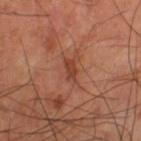Case summary:
• biopsy status · total-body-photography surveillance lesion; no biopsy
• tile lighting · cross-polarized illumination
• patient · male, in their 60s
• diameter · about 3 mm
• acquisition · total-body-photography crop, ~15 mm field of view
• location · the left thigh